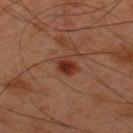workup=total-body-photography surveillance lesion; no biopsy | lighting=cross-polarized | anatomic site=the upper back | image source=~15 mm tile from a whole-body skin photo | lesion size=about 2.5 mm | subject=male, aged 43–47.